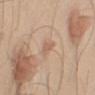Recorded during total-body skin imaging; not selected for excision or biopsy.
This is a white-light tile.
A male subject, aged 43 to 47.
The total-body-photography lesion software estimated a lesion area of about 2.5 mm², a shape eccentricity near 0.9, and a symmetry-axis asymmetry near 0.3.
A lesion tile, about 15 mm wide, cut from a 3D total-body photograph.
The lesion is on the right upper arm.
Approximately 2.5 mm at its widest.